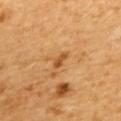Impression: The lesion was photographed on a routine skin check and not biopsied; there is no pathology result. Context: This image is a 15 mm lesion crop taken from a total-body photograph. About 2.5 mm across. A female patient aged 53–57. The lesion is on the upper back.